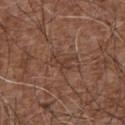Recorded during total-body skin imaging; not selected for excision or biopsy. About 3.5 mm across. The lesion-visualizer software estimated a border-irregularity index near 6/10 and internal color variation of about 2 on a 0–10 scale. The software also gave an automated nevus-likeness rating near 0 out of 100 and a lesion-detection confidence of about 60/100. The subject is a male approximately 75 years of age. Located on the chest. A region of skin cropped from a whole-body photographic capture, roughly 15 mm wide.